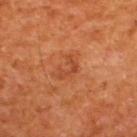Clinical impression:
No biopsy was performed on this lesion — it was imaged during a full skin examination and was not determined to be concerning.
Context:
The subject is a male in their mid- to late 60s. Automated image analysis of the tile measured an area of roughly 3 mm² and two-axis asymmetry of about 0.6. The software also gave roughly 7 lightness units darker than nearby skin and a normalized lesion–skin contrast near 5.5. The recorded lesion diameter is about 3 mm. A roughly 15 mm field-of-view crop from a total-body skin photograph. Imaged with cross-polarized lighting. The lesion is located on the upper back.